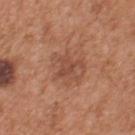workup: total-body-photography surveillance lesion; no biopsy
anatomic site: the upper back
imaging modality: ~15 mm tile from a whole-body skin photo
patient: male, roughly 55 years of age